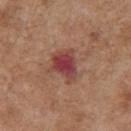Case summary:
– notes — total-body-photography surveillance lesion; no biopsy
– automated metrics — a shape eccentricity near 0.55 and two-axis asymmetry of about 0.4; a lesion–skin lightness drop of about 11 and a normalized border contrast of about 9.5; a border-irregularity rating of about 4/10 and radial color variation of about 1; lesion-presence confidence of about 100/100
– lesion size — about 4 mm
– imaging modality — total-body-photography crop, ~15 mm field of view
– body site — the chest
– patient — female, aged 73 to 77
– lighting — white-light